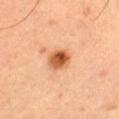Impression: The lesion was tiled from a total-body skin photograph and was not biopsied. Clinical summary: A male patient about 50 years old. Automated image analysis of the tile measured a lesion area of about 6.5 mm², a shape eccentricity near 0.5, and a symmetry-axis asymmetry near 0.15. The lesion is on the left thigh. A roughly 15 mm field-of-view crop from a total-body skin photograph. Captured under cross-polarized illumination.